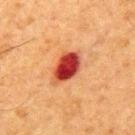An algorithmic analysis of the crop reported roughly 18 lightness units darker than nearby skin and a lesion-to-skin contrast of about 14 (normalized; higher = more distinct). A male subject in their mid- to late 60s. Measured at roughly 4.5 mm in maximum diameter. Imaged with cross-polarized lighting. A close-up tile cropped from a whole-body skin photograph, about 15 mm across. The lesion is located on the mid back.